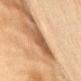follow-up: total-body-photography surveillance lesion; no biopsy
tile lighting: cross-polarized illumination
lesion size: ~5 mm (longest diameter)
body site: the abdomen
TBP lesion metrics: an area of roughly 11 mm², an eccentricity of roughly 0.85, and a symmetry-axis asymmetry near 0.35; a mean CIELAB color near L≈48 a*≈19 b*≈31, roughly 10 lightness units darker than nearby skin, and a normalized lesion–skin contrast near 7.5; an automated nevus-likeness rating near 0 out of 100 and a detector confidence of about 75 out of 100 that the crop contains a lesion
patient: female, in their 80s
imaging modality: ~15 mm tile from a whole-body skin photo